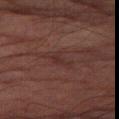Clinical impression:
Captured during whole-body skin photography for melanoma surveillance; the lesion was not biopsied.
Image and clinical context:
The total-body-photography lesion software estimated a lesion color around L≈26 a*≈17 b*≈18 in CIELAB, roughly 4 lightness units darker than nearby skin, and a normalized border contrast of about 5. The software also gave an automated nevus-likeness rating near 0 out of 100 and lesion-presence confidence of about 50/100. The patient is a male aged around 85 or older. Measured at roughly 3.5 mm in maximum diameter. A close-up tile cropped from a whole-body skin photograph, about 15 mm across. The lesion is on the right thigh. Captured under cross-polarized illumination.